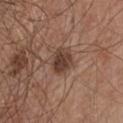This lesion was catalogued during total-body skin photography and was not selected for biopsy. Located on the chest. A close-up tile cropped from a whole-body skin photograph, about 15 mm across. The patient is a male in their mid- to late 60s.